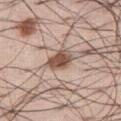<lesion>
<biopsy_status>not biopsied; imaged during a skin examination</biopsy_status>
<site>leg</site>
<patient>
  <sex>male</sex>
  <age_approx>55</age_approx>
</patient>
<lesion_size>
  <long_diameter_mm_approx>3.5</long_diameter_mm_approx>
</lesion_size>
<image>
  <source>total-body photography crop</source>
  <field_of_view_mm>15</field_of_view_mm>
</image>
<lighting>white-light</lighting>
</lesion>